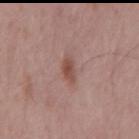Context:
This is a white-light tile. The lesion-visualizer software estimated an average lesion color of about L≈49 a*≈22 b*≈24 (CIELAB), about 9 CIELAB-L* units darker than the surrounding skin, and a normalized border contrast of about 7.5. The subject is a male aged around 55. Cropped from a total-body skin-imaging series; the visible field is about 15 mm.